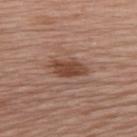Q: Automated lesion metrics?
A: a lesion area of about 6.5 mm² and a shape-asymmetry score of about 0.2 (0 = symmetric); an average lesion color of about L≈44 a*≈21 b*≈28 (CIELAB), roughly 11 lightness units darker than nearby skin, and a normalized border contrast of about 9; an automated nevus-likeness rating near 85 out of 100 and a detector confidence of about 100 out of 100 that the crop contains a lesion
Q: What is the imaging modality?
A: 15 mm crop, total-body photography
Q: Who is the patient?
A: female, in their mid- to late 60s
Q: Lesion size?
A: about 4 mm
Q: What lighting was used for the tile?
A: white-light illumination
Q: Lesion location?
A: the back